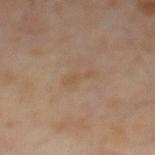No biopsy was performed on this lesion — it was imaged during a full skin examination and was not determined to be concerning.
About 3 mm across.
A close-up tile cropped from a whole-body skin photograph, about 15 mm across.
A male subject, aged 63 to 67.